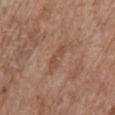Part of a total-body skin-imaging series; this lesion was reviewed on a skin check and was not flagged for biopsy.
The tile uses white-light illumination.
The lesion is located on the back.
A roughly 15 mm field-of-view crop from a total-body skin photograph.
A female subject, aged around 85 or older.
The lesion's longest dimension is about 3.5 mm.